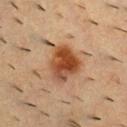Imaged with cross-polarized lighting.
The subject is a male aged around 55.
On the upper back.
A roughly 15 mm field-of-view crop from a total-body skin photograph.
An algorithmic analysis of the crop reported a lesion color around L≈42 a*≈20 b*≈31 in CIELAB, roughly 12 lightness units darker than nearby skin, and a lesion-to-skin contrast of about 10 (normalized; higher = more distinct). It also reported a color-variation rating of about 7/10 and peripheral color asymmetry of about 2.
Longest diameter approximately 6 mm.